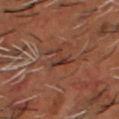No biopsy was performed on this lesion — it was imaged during a full skin examination and was not determined to be concerning. A male patient aged approximately 50. A region of skin cropped from a whole-body photographic capture, roughly 15 mm wide. The lesion is located on the head or neck.